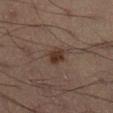Recorded during total-body skin imaging; not selected for excision or biopsy. The total-body-photography lesion software estimated a footprint of about 4.5 mm², an eccentricity of roughly 0.7, and a symmetry-axis asymmetry near 0.25. The lesion is located on the right lower leg. A male patient, approximately 60 years of age. This is a cross-polarized tile. Approximately 3 mm at its widest. A 15 mm close-up extracted from a 3D total-body photography capture.